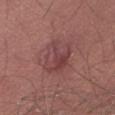follow-up: catalogued during a skin exam; not biopsied | automated lesion analysis: a lesion–skin lightness drop of about 7 and a normalized lesion–skin contrast near 6; a border-irregularity index near 3/10, a color-variation rating of about 5.5/10, and a peripheral color-asymmetry measure near 1.5; an automated nevus-likeness rating near 5 out of 100 and lesion-presence confidence of about 100/100 | patient: male, approximately 50 years of age | acquisition: total-body-photography crop, ~15 mm field of view | tile lighting: white-light illumination | body site: the right thigh | lesion size: ≈4.5 mm.